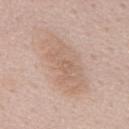Case summary:
- workup · imaged on a skin check; not biopsied
- patient · male, approximately 55 years of age
- location · the mid back
- acquisition · ~15 mm tile from a whole-body skin photo